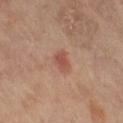Assessment: Imaged during a routine full-body skin examination; the lesion was not biopsied and no histopathology is available. Clinical summary: Longest diameter approximately 2.5 mm. The lesion is located on the abdomen. A 15 mm crop from a total-body photograph taken for skin-cancer surveillance. A female subject aged approximately 65.